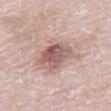Imaged during a routine full-body skin examination; the lesion was not biopsied and no histopathology is available. Captured under white-light illumination. Cropped from a total-body skin-imaging series; the visible field is about 15 mm. About 4 mm across. From the right thigh. The subject is a male roughly 80 years of age. An algorithmic analysis of the crop reported a footprint of about 12 mm², an outline eccentricity of about 0.5 (0 = round, 1 = elongated), and two-axis asymmetry of about 0.2. The analysis additionally found a classifier nevus-likeness of about 70/100 and a detector confidence of about 100 out of 100 that the crop contains a lesion.